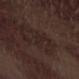Q: Was this lesion biopsied?
A: catalogued during a skin exam; not biopsied
Q: How was the tile lit?
A: white-light illumination
Q: Patient demographics?
A: male, aged around 70
Q: How was this image acquired?
A: ~15 mm tile from a whole-body skin photo
Q: Lesion location?
A: the left forearm
Q: Automated lesion metrics?
A: border irregularity of about 5.5 on a 0–10 scale, internal color variation of about 0 on a 0–10 scale, and radial color variation of about 0